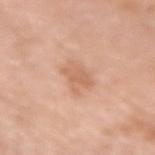| key | value |
|---|---|
| notes | total-body-photography surveillance lesion; no biopsy |
| lighting | white-light |
| diameter | ≈3.5 mm |
| patient | female, aged around 65 |
| site | the left forearm |
| imaging modality | total-body-photography crop, ~15 mm field of view |